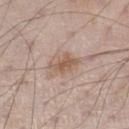Q: Was a biopsy performed?
A: catalogued during a skin exam; not biopsied
Q: What kind of image is this?
A: ~15 mm crop, total-body skin-cancer survey
Q: Where on the body is the lesion?
A: the left lower leg
Q: How large is the lesion?
A: ≈3.5 mm
Q: Patient demographics?
A: male, in their 60s